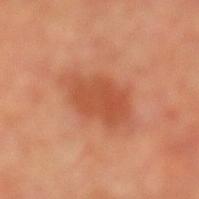biopsy status=no biopsy performed (imaged during a skin exam).